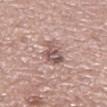Impression:
The lesion was photographed on a routine skin check and not biopsied; there is no pathology result.
Background:
On the left lower leg. Cropped from a whole-body photographic skin survey; the tile spans about 15 mm. Approximately 3.5 mm at its widest. A male patient, aged approximately 65. Automated image analysis of the tile measured a lesion area of about 7 mm², an eccentricity of roughly 0.6, and two-axis asymmetry of about 0.3. The analysis additionally found a mean CIELAB color near L≈55 a*≈18 b*≈21 and a normalized lesion–skin contrast near 8. It also reported border irregularity of about 3 on a 0–10 scale, internal color variation of about 6 on a 0–10 scale, and peripheral color asymmetry of about 2.